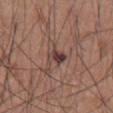Notes:
* workup — total-body-photography surveillance lesion; no biopsy
* anatomic site — the abdomen
* lesion size — about 2.5 mm
* imaging modality — total-body-photography crop, ~15 mm field of view
* patient — male, aged 53–57
* automated lesion analysis — a lesion area of about 4 mm², an eccentricity of roughly 0.8, and two-axis asymmetry of about 0.3; a lesion color around L≈39 a*≈20 b*≈19 in CIELAB, about 11 CIELAB-L* units darker than the surrounding skin, and a normalized lesion–skin contrast near 10